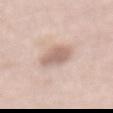Clinical impression: No biopsy was performed on this lesion — it was imaged during a full skin examination and was not determined to be concerning. Clinical summary: Cropped from a total-body skin-imaging series; the visible field is about 15 mm. Imaged with white-light lighting. About 3.5 mm across. On the abdomen. The patient is a male aged approximately 50.